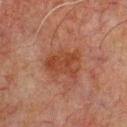Recorded during total-body skin imaging; not selected for excision or biopsy. The lesion is on the chest. The patient is a male aged approximately 60. Automated image analysis of the tile measured an area of roughly 10 mm², an outline eccentricity of about 0.7 (0 = round, 1 = elongated), and a shape-asymmetry score of about 0.35 (0 = symmetric). A lesion tile, about 15 mm wide, cut from a 3D total-body photograph. This is a cross-polarized tile.